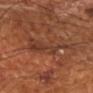This lesion was catalogued during total-body skin photography and was not selected for biopsy. Measured at roughly 5 mm in maximum diameter. A male patient aged 68 to 72. This image is a 15 mm lesion crop taken from a total-body photograph. This is a cross-polarized tile. The lesion-visualizer software estimated a mean CIELAB color near L≈36 a*≈22 b*≈28, a lesion–skin lightness drop of about 6, and a normalized lesion–skin contrast near 5.5. It also reported a border-irregularity index near 7.5/10, internal color variation of about 3.5 on a 0–10 scale, and radial color variation of about 1. The lesion is located on the left forearm.